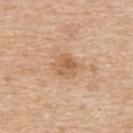Assessment: Captured during whole-body skin photography for melanoma surveillance; the lesion was not biopsied. Clinical summary: A close-up tile cropped from a whole-body skin photograph, about 15 mm across. Measured at roughly 3 mm in maximum diameter. Located on the upper back. The subject is a male roughly 60 years of age.